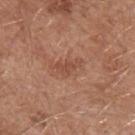Image and clinical context:
From the left upper arm. About 3 mm across. A male patient, roughly 55 years of age. This image is a 15 mm lesion crop taken from a total-body photograph.